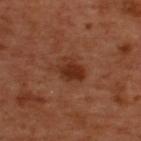biopsy_status: not biopsied; imaged during a skin examination
lighting: cross-polarized
patient:
  sex: male
  age_approx: 50
site: upper back
lesion_size:
  long_diameter_mm_approx: 3.5
image:
  source: total-body photography crop
  field_of_view_mm: 15